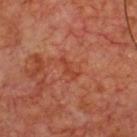<case>
<biopsy_status>not biopsied; imaged during a skin examination</biopsy_status>
<lesion_size>
  <long_diameter_mm_approx>3.0</long_diameter_mm_approx>
</lesion_size>
<image>
  <source>total-body photography crop</source>
  <field_of_view_mm>15</field_of_view_mm>
</image>
<patient>
  <sex>male</sex>
  <age_approx>65</age_approx>
</patient>
<site>chest</site>
<automated_metrics>
  <eccentricity>0.95</eccentricity>
  <cielab_L>41</cielab_L>
  <cielab_a>31</cielab_a>
  <cielab_b>33</cielab_b>
  <vs_skin_contrast_norm>5.5</vs_skin_contrast_norm>
  <lesion_detection_confidence_0_100>100</lesion_detection_confidence_0_100>
</automated_metrics>
</case>